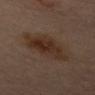The lesion was photographed on a routine skin check and not biopsied; there is no pathology result.
The lesion is on the mid back.
A region of skin cropped from a whole-body photographic capture, roughly 15 mm wide.
The total-body-photography lesion software estimated a mean CIELAB color near L≈30 a*≈16 b*≈24.